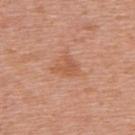biopsy_status: not biopsied; imaged during a skin examination
automated_metrics:
  nevus_likeness_0_100: 0
  lesion_detection_confidence_0_100: 100
patient:
  sex: female
  age_approx: 40
site: upper back
lighting: white-light
image:
  source: total-body photography crop
  field_of_view_mm: 15